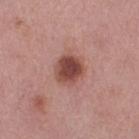Impression:
Imaged during a routine full-body skin examination; the lesion was not biopsied and no histopathology is available.